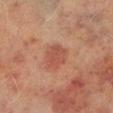notes: total-body-photography surveillance lesion; no biopsy
subject: male, in their 70s
image-analysis metrics: a lesion area of about 6.5 mm², a shape eccentricity near 0.45, and a symmetry-axis asymmetry near 0.2; a border-irregularity index near 2/10, internal color variation of about 1.5 on a 0–10 scale, and peripheral color asymmetry of about 0.5
lighting: cross-polarized illumination
anatomic site: the right lower leg
image source: ~15 mm crop, total-body skin-cancer survey